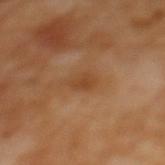The lesion was photographed on a routine skin check and not biopsied; there is no pathology result. Located on the upper back. A female patient aged 53–57. A 15 mm close-up tile from a total-body photography series done for melanoma screening. Captured under cross-polarized illumination. The total-body-photography lesion software estimated a footprint of about 3.5 mm², a shape eccentricity near 0.75, and two-axis asymmetry of about 0.25. It also reported a lesion color around L≈45 a*≈22 b*≈37 in CIELAB, roughly 7 lightness units darker than nearby skin, and a lesion-to-skin contrast of about 6 (normalized; higher = more distinct). The software also gave a within-lesion color-variation index near 1.5/10 and a peripheral color-asymmetry measure near 0.5. The software also gave lesion-presence confidence of about 100/100. The recorded lesion diameter is about 2.5 mm.